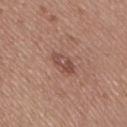workup: catalogued during a skin exam; not biopsied
body site: the leg
imaging modality: total-body-photography crop, ~15 mm field of view
patient: female, aged 38 to 42
diameter: about 3.5 mm